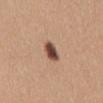The lesion was tiled from a total-body skin photograph and was not biopsied. An algorithmic analysis of the crop reported a lesion area of about 5 mm² and an outline eccentricity of about 0.7 (0 = round, 1 = elongated). It also reported a border-irregularity index near 1.5/10, a within-lesion color-variation index near 5/10, and radial color variation of about 1.5. It also reported a classifier nevus-likeness of about 100/100 and a detector confidence of about 100 out of 100 that the crop contains a lesion. From the abdomen. This image is a 15 mm lesion crop taken from a total-body photograph. About 3 mm across. A female patient, approximately 30 years of age.